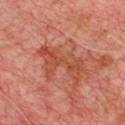Clinical impression:
Recorded during total-body skin imaging; not selected for excision or biopsy.
Context:
The lesion is located on the chest. This image is a 15 mm lesion crop taken from a total-body photograph. A male patient aged 63–67.